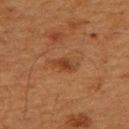Assessment: The lesion was tiled from a total-body skin photograph and was not biopsied. Background: Cropped from a whole-body photographic skin survey; the tile spans about 15 mm. The lesion is on the upper back. This is a cross-polarized tile. Measured at roughly 3 mm in maximum diameter. A male subject, in their mid-60s.